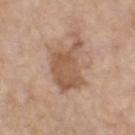workup: total-body-photography surveillance lesion; no biopsy | location: the head or neck | image-analysis metrics: an area of roughly 15 mm², a shape eccentricity near 0.75, and a symmetry-axis asymmetry near 0.4; an average lesion color of about L≈55 a*≈19 b*≈31 (CIELAB), a lesion–skin lightness drop of about 11, and a lesion-to-skin contrast of about 7.5 (normalized; higher = more distinct); a border-irregularity index near 5/10 and internal color variation of about 3 on a 0–10 scale | imaging modality: 15 mm crop, total-body photography | size: about 6 mm | patient: male, roughly 70 years of age | illumination: white-light.